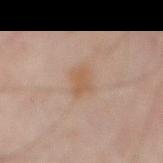Q: Was this lesion biopsied?
A: no biopsy performed (imaged during a skin exam)
Q: What lighting was used for the tile?
A: cross-polarized
Q: What is the anatomic site?
A: the abdomen
Q: What are the patient's age and sex?
A: male, in their 60s
Q: What kind of image is this?
A: 15 mm crop, total-body photography
Q: Lesion size?
A: about 2.5 mm